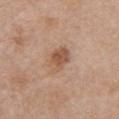Clinical impression:
Recorded during total-body skin imaging; not selected for excision or biopsy.
Image and clinical context:
About 3.5 mm across. The patient is a female aged approximately 40. Imaged with white-light lighting. Located on the chest. Automated image analysis of the tile measured a footprint of about 7 mm², a shape eccentricity near 0.7, and a shape-asymmetry score of about 0.2 (0 = symmetric). A close-up tile cropped from a whole-body skin photograph, about 15 mm across.